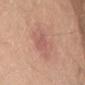Imaged during a routine full-body skin examination; the lesion was not biopsied and no histopathology is available.
Longest diameter approximately 6.5 mm.
A female patient aged around 45.
A close-up tile cropped from a whole-body skin photograph, about 15 mm across.
The lesion is on the right thigh.